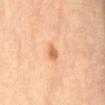Case summary:
– notes · no biopsy performed (imaged during a skin exam)
– patient · female, in their mid-60s
– illumination · cross-polarized
– acquisition · ~15 mm crop, total-body skin-cancer survey
– diameter · about 2.5 mm
– site · the chest